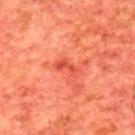Clinical impression:
Imaged during a routine full-body skin examination; the lesion was not biopsied and no histopathology is available.
Clinical summary:
A male subject approximately 65 years of age. Located on the upper back. A lesion tile, about 15 mm wide, cut from a 3D total-body photograph. An algorithmic analysis of the crop reported a footprint of about 4 mm², an eccentricity of roughly 0.9, and a symmetry-axis asymmetry near 0.35. The software also gave an average lesion color of about L≈50 a*≈40 b*≈37 (CIELAB), roughly 8 lightness units darker than nearby skin, and a lesion-to-skin contrast of about 5.5 (normalized; higher = more distinct).